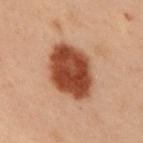Image and clinical context: The tile uses cross-polarized illumination. A male subject, roughly 55 years of age. The lesion is located on the right upper arm. About 6.5 mm across. A 15 mm crop from a total-body photograph taken for skin-cancer surveillance.